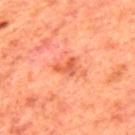Q: What is the anatomic site?
A: the mid back
Q: Illumination type?
A: cross-polarized illumination
Q: Automated lesion metrics?
A: a shape eccentricity near 0.75 and a symmetry-axis asymmetry near 0.6; a nevus-likeness score of about 0/100 and lesion-presence confidence of about 100/100
Q: Lesion size?
A: about 3 mm
Q: What are the patient's age and sex?
A: male, about 65 years old
Q: What kind of image is this?
A: 15 mm crop, total-body photography